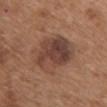{
  "biopsy_status": "not biopsied; imaged during a skin examination",
  "patient": {
    "sex": "female",
    "age_approx": 70
  },
  "site": "chest",
  "image": {
    "source": "total-body photography crop",
    "field_of_view_mm": 15
  },
  "lesion_size": {
    "long_diameter_mm_approx": 6.0
  },
  "lighting": "white-light"
}